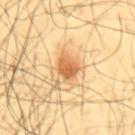Clinical impression:
The lesion was tiled from a total-body skin photograph and was not biopsied.
Clinical summary:
A male patient in their mid- to late 40s. This is a cross-polarized tile. On the mid back. About 3 mm across. This image is a 15 mm lesion crop taken from a total-body photograph.